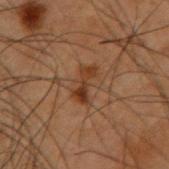site: right forearm
automated_metrics:
  eccentricity: 0.95
  cielab_L: 27
  cielab_a: 17
  cielab_b: 26
  vs_skin_darker_L: 8.0
  vs_skin_contrast_norm: 8.5
  color_variation_0_10: 2.5
  nevus_likeness_0_100: 20
  lesion_detection_confidence_0_100: 100
lighting: cross-polarized
lesion_size:
  long_diameter_mm_approx: 4.0
patient:
  sex: male
  age_approx: 50
image:
  source: total-body photography crop
  field_of_view_mm: 15